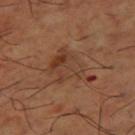Q: What is the lesion's diameter?
A: about 5 mm
Q: Where on the body is the lesion?
A: the right thigh
Q: What kind of image is this?
A: total-body-photography crop, ~15 mm field of view
Q: What did automated image analysis measure?
A: a footprint of about 14 mm²; border irregularity of about 5.5 on a 0–10 scale; a nevus-likeness score of about 0/100 and a detector confidence of about 100 out of 100 that the crop contains a lesion
Q: What lighting was used for the tile?
A: cross-polarized
Q: What are the patient's age and sex?
A: male, aged 63 to 67